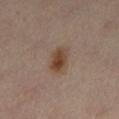This is a cross-polarized tile. On the right leg. A female patient, aged around 40. Cropped from a whole-body photographic skin survey; the tile spans about 15 mm. The total-body-photography lesion software estimated a color-variation rating of about 5/10 and a peripheral color-asymmetry measure near 1.5.